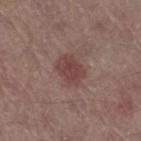Part of a total-body skin-imaging series; this lesion was reviewed on a skin check and was not flagged for biopsy.